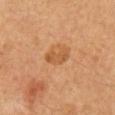Assessment:
The lesion was tiled from a total-body skin photograph and was not biopsied.
Image and clinical context:
On the mid back. A lesion tile, about 15 mm wide, cut from a 3D total-body photograph. Captured under cross-polarized illumination. A male patient, in their 60s. About 3 mm across.